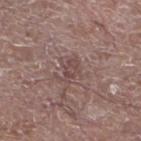Background:
On the left lower leg. Imaged with white-light lighting. This image is a 15 mm lesion crop taken from a total-body photograph. Approximately 3 mm at its widest. A male patient, in their mid-60s.